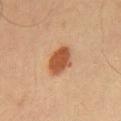No biopsy was performed on this lesion — it was imaged during a full skin examination and was not determined to be concerning.
Captured under cross-polarized illumination.
The patient is a male aged 43 to 47.
Measured at roughly 4 mm in maximum diameter.
An algorithmic analysis of the crop reported a shape eccentricity near 0.8 and a symmetry-axis asymmetry near 0.15. The software also gave roughly 14 lightness units darker than nearby skin and a lesion-to-skin contrast of about 10.5 (normalized; higher = more distinct). The analysis additionally found a border-irregularity index near 2/10 and a within-lesion color-variation index near 2.5/10. It also reported a nevus-likeness score of about 100/100 and a detector confidence of about 100 out of 100 that the crop contains a lesion.
The lesion is located on the chest.
A 15 mm close-up extracted from a 3D total-body photography capture.